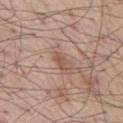{
  "biopsy_status": "not biopsied; imaged during a skin examination",
  "lighting": "white-light",
  "lesion_size": {
    "long_diameter_mm_approx": 2.5
  },
  "site": "chest",
  "automated_metrics": {
    "cielab_L": 53,
    "cielab_a": 20,
    "cielab_b": 27,
    "vs_skin_darker_L": 9.0,
    "vs_skin_contrast_norm": 6.5
  },
  "patient": {
    "sex": "male",
    "age_approx": 55
  },
  "image": {
    "source": "total-body photography crop",
    "field_of_view_mm": 15
  }
}